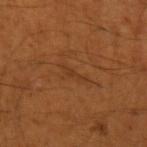Findings:
- workup — no biopsy performed (imaged during a skin exam)
- automated lesion analysis — an area of roughly 2 mm², an eccentricity of roughly 0.95, and a symmetry-axis asymmetry near 0.5; a border-irregularity index near 6.5/10, a within-lesion color-variation index near 0/10, and a peripheral color-asymmetry measure near 0; lesion-presence confidence of about 70/100
- lighting — cross-polarized
- patient — male, about 55 years old
- site — the left upper arm
- acquisition — ~15 mm crop, total-body skin-cancer survey
- size — ~3 mm (longest diameter)